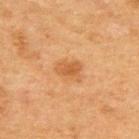<tbp_lesion>
<patient>
  <sex>male</sex>
  <age_approx>50</age_approx>
</patient>
<site>upper back</site>
<lighting>cross-polarized</lighting>
<lesion_size>
  <long_diameter_mm_approx>3.0</long_diameter_mm_approx>
</lesion_size>
<image>
  <source>total-body photography crop</source>
  <field_of_view_mm>15</field_of_view_mm>
</image>
<automated_metrics>
  <color_variation_0_10>2.0</color_variation_0_10>
  <peripheral_color_asymmetry>0.5</peripheral_color_asymmetry>
  <nevus_likeness_0_100>55</nevus_likeness_0_100>
  <lesion_detection_confidence_0_100>100</lesion_detection_confidence_0_100>
</automated_metrics>
</tbp_lesion>